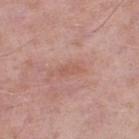Findings:
• workup · imaged on a skin check; not biopsied
• TBP lesion metrics · a mean CIELAB color near L≈57 a*≈23 b*≈27 and roughly 6 lightness units darker than nearby skin; a nevus-likeness score of about 0/100 and lesion-presence confidence of about 100/100
• body site · the left lower leg
• acquisition · 15 mm crop, total-body photography
• subject · male, in their mid- to late 50s
• illumination · white-light illumination
• lesion diameter · ~2.5 mm (longest diameter)